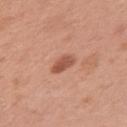<case>
  <biopsy_status>not biopsied; imaged during a skin examination</biopsy_status>
  <image>
    <source>total-body photography crop</source>
    <field_of_view_mm>15</field_of_view_mm>
  </image>
  <patient>
    <sex>female</sex>
    <age_approx>50</age_approx>
  </patient>
  <site>left upper arm</site>
  <automated_metrics>
    <cielab_L>54</cielab_L>
    <cielab_a>26</cielab_a>
    <cielab_b>31</cielab_b>
    <vs_skin_contrast_norm>8.0</vs_skin_contrast_norm>
    <border_irregularity_0_10>2.0</border_irregularity_0_10>
    <peripheral_color_asymmetry>1.0</peripheral_color_asymmetry>
    <lesion_detection_confidence_0_100>100</lesion_detection_confidence_0_100>
  </automated_metrics>
</case>